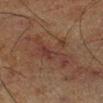Assessment:
Part of a total-body skin-imaging series; this lesion was reviewed on a skin check and was not flagged for biopsy.
Background:
The lesion is on the right lower leg. An algorithmic analysis of the crop reported a color-variation rating of about 4/10 and radial color variation of about 1.5. The analysis additionally found a classifier nevus-likeness of about 0/100 and lesion-presence confidence of about 90/100. Cropped from a whole-body photographic skin survey; the tile spans about 15 mm. The subject is a male approximately 60 years of age. This is a cross-polarized tile. The lesion's longest dimension is about 8 mm.